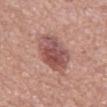The lesion was photographed on a routine skin check and not biopsied; there is no pathology result. A 15 mm close-up tile from a total-body photography series done for melanoma screening. Located on the abdomen. Automated image analysis of the tile measured a border-irregularity rating of about 1.5/10. And it measured a nevus-likeness score of about 60/100 and lesion-presence confidence of about 100/100. The recorded lesion diameter is about 5.5 mm. This is a white-light tile. The subject is a male aged 73 to 77.